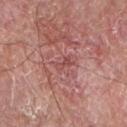| field | value |
|---|---|
| workup | imaged on a skin check; not biopsied |
| diameter | ≈2.5 mm |
| anatomic site | the right forearm |
| subject | male, aged approximately 60 |
| image | 15 mm crop, total-body photography |